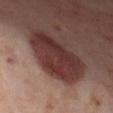follow-up = total-body-photography surveillance lesion; no biopsy | image-analysis metrics = a classifier nevus-likeness of about 60/100 and a lesion-detection confidence of about 85/100 | lighting = cross-polarized illumination | diameter = about 8 mm | patient = female, approximately 55 years of age | acquisition = 15 mm crop, total-body photography | site = the leg.